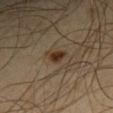Q: Was a biopsy performed?
A: total-body-photography surveillance lesion; no biopsy
Q: What is the anatomic site?
A: the arm
Q: What kind of image is this?
A: ~15 mm crop, total-body skin-cancer survey
Q: How large is the lesion?
A: about 2.5 mm
Q: Who is the patient?
A: male, aged 63 to 67
Q: What lighting was used for the tile?
A: cross-polarized illumination